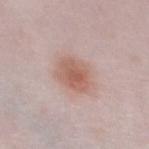  biopsy_status: not biopsied; imaged during a skin examination
  patient:
    sex: female
    age_approx: 65
  lighting: white-light
  image:
    source: total-body photography crop
    field_of_view_mm: 15
  site: chest
  automated_metrics:
    area_mm2_approx: 11.0
    eccentricity: 0.4
    shape_asymmetry: 0.15
    cielab_L: 59
    cielab_a: 20
    cielab_b: 26
    vs_skin_darker_L: 10.0
    vs_skin_contrast_norm: 7.5
    border_irregularity_0_10: 2.0
  lesion_size:
    long_diameter_mm_approx: 4.0